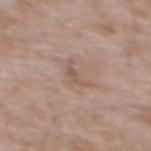Findings:
• workup: catalogued during a skin exam; not biopsied
• patient: male, in their 50s
• image: ~15 mm crop, total-body skin-cancer survey
• anatomic site: the mid back